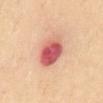workup=imaged on a skin check; not biopsied
imaging modality=total-body-photography crop, ~15 mm field of view
illumination=cross-polarized illumination
anatomic site=the abdomen
subject=male, approximately 70 years of age
image-analysis metrics=an area of roughly 12 mm²; an average lesion color of about L≈57 a*≈33 b*≈27 (CIELAB), about 16 CIELAB-L* units darker than the surrounding skin, and a normalized lesion–skin contrast near 10.5; a border-irregularity index near 1/10, internal color variation of about 8 on a 0–10 scale, and radial color variation of about 2.5; an automated nevus-likeness rating near 0 out of 100